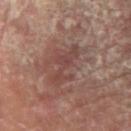biopsy status=imaged on a skin check; not biopsied
imaging modality=~15 mm crop, total-body skin-cancer survey
lesion size=≈6 mm
subject=male, roughly 70 years of age
illumination=cross-polarized
TBP lesion metrics=a shape eccentricity near 0.95 and a symmetry-axis asymmetry near 0.5; a within-lesion color-variation index near 1.5/10 and peripheral color asymmetry of about 0.5
site=the right forearm